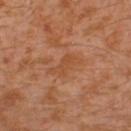Captured during whole-body skin photography for melanoma surveillance; the lesion was not biopsied.
A 15 mm close-up extracted from a 3D total-body photography capture.
The tile uses cross-polarized illumination.
On the leg.
An algorithmic analysis of the crop reported a border-irregularity rating of about 5.5/10, internal color variation of about 2 on a 0–10 scale, and a peripheral color-asymmetry measure near 0.5. And it measured a classifier nevus-likeness of about 0/100 and lesion-presence confidence of about 100/100.
A male subject approximately 30 years of age.